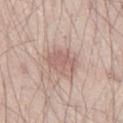biopsy_status: not biopsied; imaged during a skin examination
patient:
  sex: male
  age_approx: 40
lighting: white-light
image:
  source: total-body photography crop
  field_of_view_mm: 15
site: right thigh
lesion_size:
  long_diameter_mm_approx: 3.5